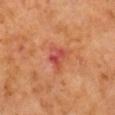Impression:
No biopsy was performed on this lesion — it was imaged during a full skin examination and was not determined to be concerning.
Acquisition and patient details:
From the right arm. The patient is a male aged 58 to 62. A 15 mm close-up tile from a total-body photography series done for melanoma screening.